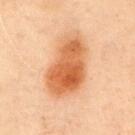{"biopsy_status": "not biopsied; imaged during a skin examination", "image": {"source": "total-body photography crop", "field_of_view_mm": 15}, "patient": {"sex": "male", "age_approx": 55}, "site": "chest"}